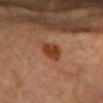{
  "biopsy_status": "not biopsied; imaged during a skin examination",
  "site": "chest",
  "image": {
    "source": "total-body photography crop",
    "field_of_view_mm": 15
  },
  "automated_metrics": {
    "cielab_L": 42,
    "cielab_a": 26,
    "cielab_b": 37,
    "vs_skin_darker_L": 11.0,
    "vs_skin_contrast_norm": 9.5,
    "border_irregularity_0_10": 2.0,
    "color_variation_0_10": 2.5,
    "peripheral_color_asymmetry": 1.0,
    "lesion_detection_confidence_0_100": 100
  },
  "patient": {
    "sex": "female",
    "age_approx": 60
  }
}